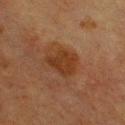This lesion was catalogued during total-body skin photography and was not selected for biopsy. A male subject, in their mid-70s. The lesion-visualizer software estimated a lesion color around L≈29 a*≈19 b*≈28 in CIELAB, about 7 CIELAB-L* units darker than the surrounding skin, and a normalized border contrast of about 8. It also reported border irregularity of about 3 on a 0–10 scale and a peripheral color-asymmetry measure near 0.5. The software also gave an automated nevus-likeness rating near 45 out of 100. Approximately 4 mm at its widest. The lesion is on the front of the torso. A close-up tile cropped from a whole-body skin photograph, about 15 mm across. Captured under cross-polarized illumination.